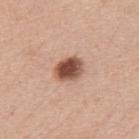Q: Is there a histopathology result?
A: total-body-photography surveillance lesion; no biopsy
Q: What is the anatomic site?
A: the left upper arm
Q: What kind of image is this?
A: ~15 mm tile from a whole-body skin photo
Q: How was the tile lit?
A: white-light
Q: Who is the patient?
A: female, in their mid- to late 40s
Q: Automated lesion metrics?
A: a classifier nevus-likeness of about 100/100 and a detector confidence of about 100 out of 100 that the crop contains a lesion
Q: How large is the lesion?
A: ≈3.5 mm